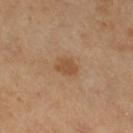workup — no biopsy performed (imaged during a skin exam) | diameter — ~3 mm (longest diameter) | automated metrics — a footprint of about 5 mm², an outline eccentricity of about 0.65 (0 = round, 1 = elongated), and two-axis asymmetry of about 0.2; a lesion-detection confidence of about 100/100 | subject — female, in their mid-50s | image source — total-body-photography crop, ~15 mm field of view | site — the right thigh.